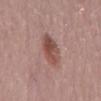| field | value |
|---|---|
| follow-up | total-body-photography surveillance lesion; no biopsy |
| tile lighting | white-light |
| image | 15 mm crop, total-body photography |
| patient | female, aged 63–67 |
| site | the mid back |
| lesion size | ≈4 mm |